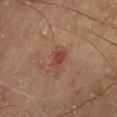Impression: Captured during whole-body skin photography for melanoma surveillance; the lesion was not biopsied.